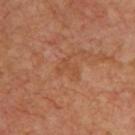biopsy_status: not biopsied; imaged during a skin examination
site: upper back
image:
  source: total-body photography crop
  field_of_view_mm: 15
patient:
  sex: male
  age_approx: 65